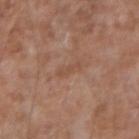biopsy status: no biopsy performed (imaged during a skin exam)
image: ~15 mm crop, total-body skin-cancer survey
automated lesion analysis: border irregularity of about 5 on a 0–10 scale, a within-lesion color-variation index near 0/10, and a peripheral color-asymmetry measure near 0; a classifier nevus-likeness of about 0/100 and lesion-presence confidence of about 80/100
location: the arm
tile lighting: white-light illumination
lesion size: ~3 mm (longest diameter)
patient: male, about 55 years old